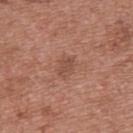Notes:
• diameter · ≈2.5 mm
• subject · female, in their 40s
• automated lesion analysis · an area of roughly 4.5 mm², an outline eccentricity of about 0.65 (0 = round, 1 = elongated), and a symmetry-axis asymmetry near 0.2; about 7 CIELAB-L* units darker than the surrounding skin and a lesion-to-skin contrast of about 5.5 (normalized; higher = more distinct)
• location · the upper back
• lighting · white-light illumination
• image · total-body-photography crop, ~15 mm field of view